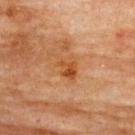The lesion was photographed on a routine skin check and not biopsied; there is no pathology result.
A female patient approximately 80 years of age.
Imaged with cross-polarized lighting.
This image is a 15 mm lesion crop taken from a total-body photograph.
The lesion is on the upper back.
Automated image analysis of the tile measured an area of roughly 5.5 mm², an eccentricity of roughly 0.65, and two-axis asymmetry of about 0.35. And it measured a mean CIELAB color near L≈44 a*≈23 b*≈37 and a normalized lesion–skin contrast near 7.